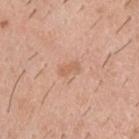Imaged during a routine full-body skin examination; the lesion was not biopsied and no histopathology is available. A male subject, in their 40s. The lesion is located on the upper back. A 15 mm close-up extracted from a 3D total-body photography capture. The total-body-photography lesion software estimated a mean CIELAB color near L≈62 a*≈22 b*≈32 and roughly 7 lightness units darker than nearby skin. And it measured a border-irregularity rating of about 2.5/10, internal color variation of about 1.5 on a 0–10 scale, and peripheral color asymmetry of about 0.5. The analysis additionally found a lesion-detection confidence of about 100/100. This is a white-light tile. Approximately 3 mm at its widest.